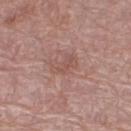Q: Patient demographics?
A: female, roughly 65 years of age
Q: Automated lesion metrics?
A: internal color variation of about 2 on a 0–10 scale and radial color variation of about 0.5; an automated nevus-likeness rating near 0 out of 100 and lesion-presence confidence of about 100/100
Q: Lesion location?
A: the right thigh
Q: How was the tile lit?
A: white-light illumination
Q: How large is the lesion?
A: ~3.5 mm (longest diameter)
Q: What is the imaging modality?
A: total-body-photography crop, ~15 mm field of view